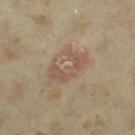patient — female, aged 33–37; site — the left thigh; acquisition — total-body-photography crop, ~15 mm field of view.